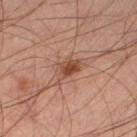notes: total-body-photography surveillance lesion; no biopsy
imaging modality: ~15 mm crop, total-body skin-cancer survey
tile lighting: cross-polarized illumination
patient: male, about 45 years old
size: ≈3 mm
site: the left lower leg